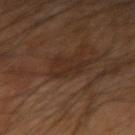{
  "image": {
    "source": "total-body photography crop",
    "field_of_view_mm": 15
  },
  "lighting": "cross-polarized",
  "automated_metrics": {
    "cielab_L": 26,
    "cielab_a": 16,
    "cielab_b": 24,
    "vs_skin_darker_L": 5.0,
    "vs_skin_contrast_norm": 6.0,
    "border_irregularity_0_10": 6.0,
    "peripheral_color_asymmetry": 0.5,
    "nevus_likeness_0_100": 5,
    "lesion_detection_confidence_0_100": 90
  },
  "patient": {
    "sex": "male",
    "age_approx": 60
  },
  "site": "left forearm",
  "lesion_size": {
    "long_diameter_mm_approx": 4.0
  }
}